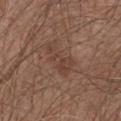Impression:
No biopsy was performed on this lesion — it was imaged during a full skin examination and was not determined to be concerning.
Acquisition and patient details:
A lesion tile, about 15 mm wide, cut from a 3D total-body photograph. The patient is a male in their mid- to late 60s. From the front of the torso.